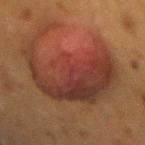The total-body-photography lesion software estimated a lesion area of about 50 mm² and an eccentricity of roughly 0.6. And it measured border irregularity of about 8.5 on a 0–10 scale, internal color variation of about 7 on a 0–10 scale, and a peripheral color-asymmetry measure near 2.
From the mid back.
The lesion's longest dimension is about 10.5 mm.
The tile uses cross-polarized illumination.
A female subject about 50 years old.
A region of skin cropped from a whole-body photographic capture, roughly 15 mm wide.